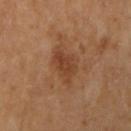Imaged during a routine full-body skin examination; the lesion was not biopsied and no histopathology is available.
This is a cross-polarized tile.
Measured at roughly 4 mm in maximum diameter.
Cropped from a total-body skin-imaging series; the visible field is about 15 mm.
On the right upper arm.
The subject is a female roughly 60 years of age.
The lesion-visualizer software estimated a mean CIELAB color near L≈41 a*≈22 b*≈33 and a lesion-to-skin contrast of about 7 (normalized; higher = more distinct).